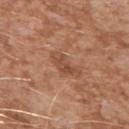Clinical impression:
Recorded during total-body skin imaging; not selected for excision or biopsy.
Clinical summary:
An algorithmic analysis of the crop reported an area of roughly 4.5 mm², an eccentricity of roughly 0.9, and a symmetry-axis asymmetry near 0.5. The analysis additionally found a mean CIELAB color near L≈48 a*≈23 b*≈31, a lesion–skin lightness drop of about 8, and a normalized lesion–skin contrast near 6. The analysis additionally found a border-irregularity rating of about 6/10, a within-lesion color-variation index near 2/10, and peripheral color asymmetry of about 0.5. A 15 mm close-up tile from a total-body photography series done for melanoma screening. This is a white-light tile. The lesion is on the left upper arm. A male subject about 45 years old.